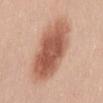The lesion was tiled from a total-body skin photograph and was not biopsied.
A female subject, approximately 30 years of age.
An algorithmic analysis of the crop reported an area of roughly 31 mm² and a symmetry-axis asymmetry near 0.1. The analysis additionally found a lesion color around L≈57 a*≈24 b*≈31 in CIELAB, a lesion–skin lightness drop of about 15, and a lesion-to-skin contrast of about 9.5 (normalized; higher = more distinct). And it measured an automated nevus-likeness rating near 100 out of 100 and a detector confidence of about 100 out of 100 that the crop contains a lesion.
A lesion tile, about 15 mm wide, cut from a 3D total-body photograph.
The lesion is located on the front of the torso.
Captured under white-light illumination.